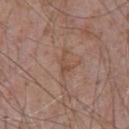Recorded during total-body skin imaging; not selected for excision or biopsy. This is a white-light tile. A 15 mm crop from a total-body photograph taken for skin-cancer surveillance. On the chest. The lesion-visualizer software estimated a within-lesion color-variation index near 0/10 and peripheral color asymmetry of about 0. The patient is a male in their 70s.